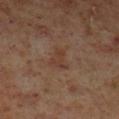automated lesion analysis: a mean CIELAB color near L≈34 a*≈19 b*≈24; a within-lesion color-variation index near 0.5/10 and radial color variation of about 0 | body site: the left lower leg | patient: male, about 60 years old | diameter: ~2.5 mm (longest diameter) | acquisition: ~15 mm crop, total-body skin-cancer survey | tile lighting: cross-polarized.